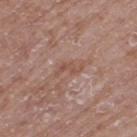No biopsy was performed on this lesion — it was imaged during a full skin examination and was not determined to be concerning. Longest diameter approximately 3 mm. Cropped from a whole-body photographic skin survey; the tile spans about 15 mm. Imaged with white-light lighting. A male subject aged around 60. The lesion is located on the right thigh.